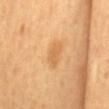follow-up: imaged on a skin check; not biopsied | image: ~15 mm crop, total-body skin-cancer survey | lesion diameter: about 3.5 mm | image-analysis metrics: a nevus-likeness score of about 5/100 | body site: the mid back | subject: male, roughly 65 years of age.